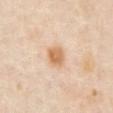The total-body-photography lesion software estimated a lesion area of about 6 mm² and two-axis asymmetry of about 0.2. It also reported an average lesion color of about L≈67 a*≈20 b*≈37 (CIELAB), about 11 CIELAB-L* units darker than the surrounding skin, and a lesion-to-skin contrast of about 8.5 (normalized; higher = more distinct). The analysis additionally found a border-irregularity rating of about 1.5/10, a color-variation rating of about 3/10, and radial color variation of about 1. The software also gave a nevus-likeness score of about 95/100 and lesion-presence confidence of about 100/100. A male subject aged 63–67. A roughly 15 mm field-of-view crop from a total-body skin photograph. Located on the abdomen. Approximately 2.5 mm at its widest.